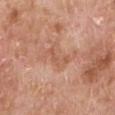{"biopsy_status": "not biopsied; imaged during a skin examination", "lesion_size": {"long_diameter_mm_approx": 3.5}, "patient": {"sex": "male", "age_approx": 80}, "image": {"source": "total-body photography crop", "field_of_view_mm": 15}, "automated_metrics": {"area_mm2_approx": 3.5, "eccentricity": 0.9, "shape_asymmetry": 0.7, "border_irregularity_0_10": 8.0, "color_variation_0_10": 0.0}, "site": "left lower leg"}